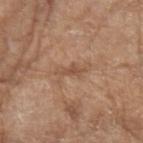* notes: imaged on a skin check; not biopsied
* subject: female, in their mid- to late 70s
* site: the right forearm
* acquisition: total-body-photography crop, ~15 mm field of view